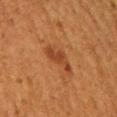The lesion was tiled from a total-body skin photograph and was not biopsied.
Cropped from a total-body skin-imaging series; the visible field is about 15 mm.
On the right upper arm.
The patient is a female approximately 50 years of age.